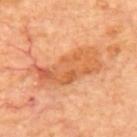| field | value |
|---|---|
| notes | catalogued during a skin exam; not biopsied |
| acquisition | ~15 mm crop, total-body skin-cancer survey |
| anatomic site | the mid back |
| subject | about 65 years old |
| size | about 8 mm |